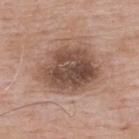<record>
<biopsy_status>not biopsied; imaged during a skin examination</biopsy_status>
<patient>
  <sex>male</sex>
  <age_approx>60</age_approx>
</patient>
<automated_metrics>
  <area_mm2_approx>31.0</area_mm2_approx>
  <shape_asymmetry>0.2</shape_asymmetry>
  <border_irregularity_0_10>2.5</border_irregularity_0_10>
  <color_variation_0_10>7.0</color_variation_0_10>
  <peripheral_color_asymmetry>2.5</peripheral_color_asymmetry>
</automated_metrics>
<image>
  <source>total-body photography crop</source>
  <field_of_view_mm>15</field_of_view_mm>
</image>
<lighting>white-light</lighting>
<site>upper back</site>
<lesion_size>
  <long_diameter_mm_approx>7.0</long_diameter_mm_approx>
</lesion_size>
</record>